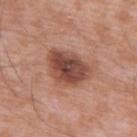The lesion is on the front of the torso. The subject is a male aged around 60. A lesion tile, about 15 mm wide, cut from a 3D total-body photograph.